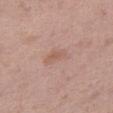No biopsy was performed on this lesion — it was imaged during a full skin examination and was not determined to be concerning.
A 15 mm close-up extracted from a 3D total-body photography capture.
A female subject roughly 40 years of age.
On the right thigh.